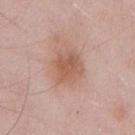The lesion was photographed on a routine skin check and not biopsied; there is no pathology result. From the chest. Cropped from a whole-body photographic skin survey; the tile spans about 15 mm. Longest diameter approximately 4 mm. The tile uses white-light illumination. The lesion-visualizer software estimated a footprint of about 10 mm² and two-axis asymmetry of about 0.2. The software also gave a border-irregularity index near 2.5/10, a color-variation rating of about 3.5/10, and radial color variation of about 1. The software also gave an automated nevus-likeness rating near 20 out of 100 and lesion-presence confidence of about 100/100. The subject is a male aged 53 to 57.